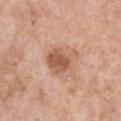{
  "biopsy_status": "not biopsied; imaged during a skin examination",
  "lesion_size": {
    "long_diameter_mm_approx": 4.0
  },
  "patient": {
    "sex": "female",
    "age_approx": 75
  },
  "image": {
    "source": "total-body photography crop",
    "field_of_view_mm": 15
  },
  "site": "front of the torso"
}